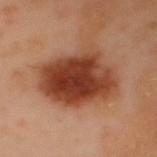Q: Was this lesion biopsied?
A: catalogued during a skin exam; not biopsied
Q: Where on the body is the lesion?
A: the mid back
Q: What kind of image is this?
A: ~15 mm crop, total-body skin-cancer survey
Q: What are the patient's age and sex?
A: female, approximately 50 years of age
Q: Illumination type?
A: cross-polarized